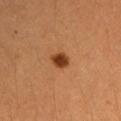Impression: No biopsy was performed on this lesion — it was imaged during a full skin examination and was not determined to be concerning. Background: A female subject aged 38–42. A 15 mm close-up tile from a total-body photography series done for melanoma screening. The lesion is located on the left upper arm. Automated tile analysis of the lesion measured a lesion area of about 4 mm² and two-axis asymmetry of about 0.15. It also reported a border-irregularity rating of about 1.5/10, internal color variation of about 2 on a 0–10 scale, and peripheral color asymmetry of about 0.5.